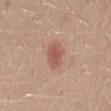- lesion size: ~3 mm (longest diameter)
- anatomic site: the mid back
- patient: female, in their 40s
- image-analysis metrics: a footprint of about 6 mm², an outline eccentricity of about 0.7 (0 = round, 1 = elongated), and a symmetry-axis asymmetry near 0.2; a nevus-likeness score of about 90/100 and a detector confidence of about 100 out of 100 that the crop contains a lesion
- acquisition: 15 mm crop, total-body photography